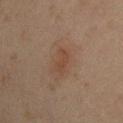workup: imaged on a skin check; not biopsied
TBP lesion metrics: a shape eccentricity near 0.85; an average lesion color of about L≈39 a*≈17 b*≈26 (CIELAB), roughly 5 lightness units darker than nearby skin, and a lesion-to-skin contrast of about 5.5 (normalized; higher = more distinct); a classifier nevus-likeness of about 35/100 and a lesion-detection confidence of about 100/100
lesion size: about 3 mm
tile lighting: cross-polarized
acquisition: 15 mm crop, total-body photography
body site: the chest
patient: male, aged around 50